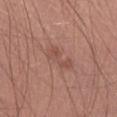biopsy status — no biopsy performed (imaged during a skin exam) | size — about 4 mm | anatomic site — the left lower leg | imaging modality — 15 mm crop, total-body photography | patient — male, about 55 years old | image-analysis metrics — a border-irregularity index near 5.5/10, a within-lesion color-variation index near 2/10, and radial color variation of about 1; a classifier nevus-likeness of about 0/100 | tile lighting — white-light.